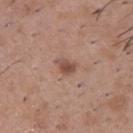A close-up tile cropped from a whole-body skin photograph, about 15 mm across.
On the upper back.
Imaged with white-light lighting.
Approximately 2.5 mm at its widest.
A male patient roughly 50 years of age.